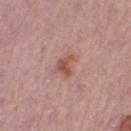biopsy status: imaged on a skin check; not biopsied | lesion diameter: ~2.5 mm (longest diameter) | anatomic site: the left thigh | subject: female, aged 48–52 | lighting: white-light illumination | image: ~15 mm tile from a whole-body skin photo.